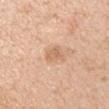Assessment: This lesion was catalogued during total-body skin photography and was not selected for biopsy. Context: Automated image analysis of the tile measured a lesion color around L≈66 a*≈20 b*≈35 in CIELAB and about 8 CIELAB-L* units darker than the surrounding skin. It also reported border irregularity of about 2.5 on a 0–10 scale, a color-variation rating of about 2.5/10, and a peripheral color-asymmetry measure near 1. It also reported a nevus-likeness score of about 10/100 and a detector confidence of about 100 out of 100 that the crop contains a lesion. A region of skin cropped from a whole-body photographic capture, roughly 15 mm wide. This is a white-light tile. Measured at roughly 2.5 mm in maximum diameter. From the left upper arm. A male patient, approximately 55 years of age.